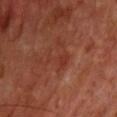A 15 mm close-up tile from a total-body photography series done for melanoma screening. The recorded lesion diameter is about 3.5 mm. A male subject roughly 65 years of age. The tile uses cross-polarized illumination.